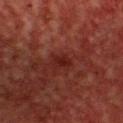{"biopsy_status": "not biopsied; imaged during a skin examination", "patient": {"sex": "male", "age_approx": 50}, "site": "chest", "image": {"source": "total-body photography crop", "field_of_view_mm": 15}}